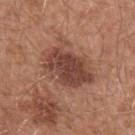Clinical impression:
Part of a total-body skin-imaging series; this lesion was reviewed on a skin check and was not flagged for biopsy.
Context:
The lesion's longest dimension is about 6.5 mm. The subject is a male roughly 55 years of age. Located on the left upper arm. The tile uses white-light illumination. Cropped from a whole-body photographic skin survey; the tile spans about 15 mm.